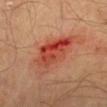patient — male, about 40 years old
anatomic site — the left lower leg
tile lighting — cross-polarized
lesion diameter — ~7 mm (longest diameter)
image — 15 mm crop, total-body photography